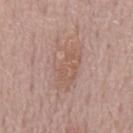Captured during whole-body skin photography for melanoma surveillance; the lesion was not biopsied.
The patient is a male about 75 years old.
On the mid back.
The tile uses white-light illumination.
A close-up tile cropped from a whole-body skin photograph, about 15 mm across.